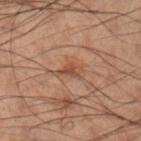biopsy status — imaged on a skin check; not biopsied
lesion diameter — ≈3.5 mm
lighting — cross-polarized illumination
site — the right lower leg
patient — male, roughly 50 years of age
imaging modality — total-body-photography crop, ~15 mm field of view